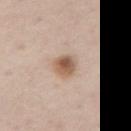No biopsy was performed on this lesion — it was imaged during a full skin examination and was not determined to be concerning.
This image is a 15 mm lesion crop taken from a total-body photograph.
Located on the right thigh.
The recorded lesion diameter is about 3.5 mm.
A male patient aged 73–77.